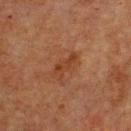Assessment: The lesion was tiled from a total-body skin photograph and was not biopsied. Image and clinical context: The patient is a male in their mid-70s. A 15 mm close-up extracted from a 3D total-body photography capture. The lesion's longest dimension is about 3.5 mm. On the chest. An algorithmic analysis of the crop reported a lesion color around L≈32 a*≈21 b*≈29 in CIELAB, roughly 6 lightness units darker than nearby skin, and a lesion-to-skin contrast of about 6.5 (normalized; higher = more distinct). And it measured border irregularity of about 4 on a 0–10 scale, internal color variation of about 1.5 on a 0–10 scale, and a peripheral color-asymmetry measure near 0. The software also gave a nevus-likeness score of about 0/100 and a detector confidence of about 100 out of 100 that the crop contains a lesion.